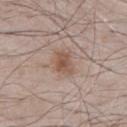{"biopsy_status": "not biopsied; imaged during a skin examination", "patient": {"sex": "male", "age_approx": 65}, "lesion_size": {"long_diameter_mm_approx": 3.5}, "site": "chest", "image": {"source": "total-body photography crop", "field_of_view_mm": 15}}